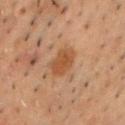Impression: This lesion was catalogued during total-body skin photography and was not selected for biopsy. Image and clinical context: The tile uses cross-polarized illumination. A 15 mm crop from a total-body photograph taken for skin-cancer surveillance. Located on the front of the torso. A male patient, about 50 years old.